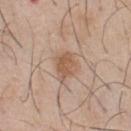Findings:
* workup · imaged on a skin check; not biopsied
* location · the front of the torso
* subject · male, about 45 years old
* imaging modality · 15 mm crop, total-body photography
* TBP lesion metrics · roughly 9 lightness units darker than nearby skin; a border-irregularity rating of about 3/10 and internal color variation of about 2.5 on a 0–10 scale; a nevus-likeness score of about 60/100 and a lesion-detection confidence of about 100/100
* lesion size · about 3.5 mm